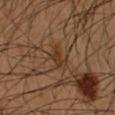The lesion was photographed on a routine skin check and not biopsied; there is no pathology result.
Measured at roughly 2.5 mm in maximum diameter.
The patient is a male in their mid- to late 50s.
A 15 mm close-up tile from a total-body photography series done for melanoma screening.
The lesion is on the left forearm.
An algorithmic analysis of the crop reported a footprint of about 4 mm² and a shape eccentricity near 0.7. And it measured border irregularity of about 3 on a 0–10 scale, internal color variation of about 1.5 on a 0–10 scale, and peripheral color asymmetry of about 0.5. The software also gave an automated nevus-likeness rating near 0 out of 100 and a lesion-detection confidence of about 100/100.
This is a cross-polarized tile.